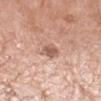Case summary:
* biopsy status — no biopsy performed (imaged during a skin exam)
* imaging modality — ~15 mm crop, total-body skin-cancer survey
* body site — the right forearm
* subject — female, aged 73 to 77